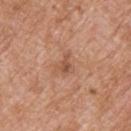Captured during whole-body skin photography for melanoma surveillance; the lesion was not biopsied.
About 3 mm across.
This image is a 15 mm lesion crop taken from a total-body photograph.
Automated image analysis of the tile measured an outline eccentricity of about 0.65 (0 = round, 1 = elongated). The software also gave a mean CIELAB color near L≈54 a*≈23 b*≈32, about 8 CIELAB-L* units darker than the surrounding skin, and a lesion-to-skin contrast of about 6 (normalized; higher = more distinct). It also reported a border-irregularity index near 5.5/10 and radial color variation of about 1.5.
The tile uses white-light illumination.
The subject is a male aged approximately 80.
On the upper back.